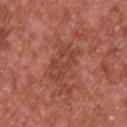Assessment: No biopsy was performed on this lesion — it was imaged during a full skin examination and was not determined to be concerning. Background: Located on the back. The patient is a male in their mid-60s. The lesion's longest dimension is about 4.5 mm. A 15 mm close-up tile from a total-body photography series done for melanoma screening. The tile uses white-light illumination.